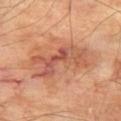follow-up=catalogued during a skin exam; not biopsied | automated metrics=a color-variation rating of about 7.5/10 and radial color variation of about 2; a classifier nevus-likeness of about 10/100 and a lesion-detection confidence of about 100/100 | patient=male, roughly 70 years of age | illumination=cross-polarized | body site=the left thigh | size=~7 mm (longest diameter) | image source=~15 mm tile from a whole-body skin photo.